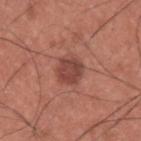Case summary:
* workup — catalogued during a skin exam; not biopsied
* image-analysis metrics — a border-irregularity rating of about 1.5/10, a color-variation rating of about 3/10, and radial color variation of about 1; a nevus-likeness score of about 90/100
* imaging modality — ~15 mm tile from a whole-body skin photo
* site — the upper back
* patient — male, roughly 35 years of age